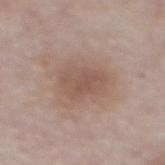Impression:
The lesion was photographed on a routine skin check and not biopsied; there is no pathology result.
Acquisition and patient details:
A male patient about 55 years old. The recorded lesion diameter is about 4.5 mm. The lesion is on the front of the torso. This is a white-light tile. Automated image analysis of the tile measured a mean CIELAB color near L≈53 a*≈17 b*≈25, a lesion–skin lightness drop of about 8, and a normalized lesion–skin contrast near 5.5. A 15 mm crop from a total-body photograph taken for skin-cancer surveillance.